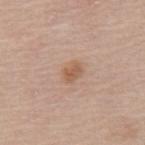acquisition: total-body-photography crop, ~15 mm field of view | location: the mid back | patient: female, aged 63–67.